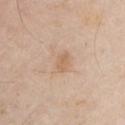Case summary:
- notes — catalogued during a skin exam; not biopsied
- acquisition — ~15 mm tile from a whole-body skin photo
- patient — male, aged around 65
- location — the chest
- lighting — white-light illumination